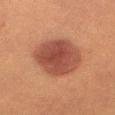workup: no biopsy performed (imaged during a skin exam) | subject: female, aged 53–57 | lesion diameter: ~6 mm (longest diameter) | lighting: cross-polarized | imaging modality: ~15 mm crop, total-body skin-cancer survey | automated lesion analysis: a shape eccentricity near 0.55; a border-irregularity rating of about 1/10 and a within-lesion color-variation index near 3.5/10 | anatomic site: the leg.